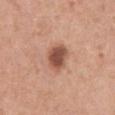Q: Illumination type?
A: white-light
Q: How large is the lesion?
A: about 4 mm
Q: Where on the body is the lesion?
A: the chest
Q: What kind of image is this?
A: total-body-photography crop, ~15 mm field of view
Q: Who is the patient?
A: male, roughly 55 years of age